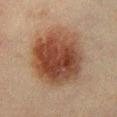notes: catalogued during a skin exam; not biopsied
location: the chest
image source: ~15 mm crop, total-body skin-cancer survey
lighting: cross-polarized illumination
subject: male, aged around 50
lesion size: ≈9 mm